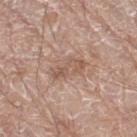The lesion was photographed on a routine skin check and not biopsied; there is no pathology result. This is a white-light tile. The lesion is on the left leg. A region of skin cropped from a whole-body photographic capture, roughly 15 mm wide. An algorithmic analysis of the crop reported a border-irregularity index near 5.5/10, a within-lesion color-variation index near 2.5/10, and peripheral color asymmetry of about 0.5. It also reported an automated nevus-likeness rating near 0 out of 100 and a lesion-detection confidence of about 95/100. The patient is a male aged around 80. The recorded lesion diameter is about 4 mm.